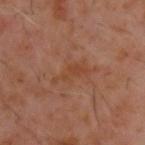lighting: cross-polarized
patient:
  sex: male
  age_approx: 60
site: upper back
lesion_size:
  long_diameter_mm_approx: 4.5
image:
  source: total-body photography crop
  field_of_view_mm: 15